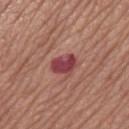Part of a total-body skin-imaging series; this lesion was reviewed on a skin check and was not flagged for biopsy.
Located on the right thigh.
The patient is a female aged 63–67.
Cropped from a whole-body photographic skin survey; the tile spans about 15 mm.